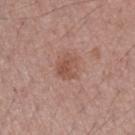No biopsy was performed on this lesion — it was imaged during a full skin examination and was not determined to be concerning. The lesion is located on the left forearm. The recorded lesion diameter is about 2.5 mm. A close-up tile cropped from a whole-body skin photograph, about 15 mm across. A female patient, in their mid-50s. The tile uses white-light illumination.